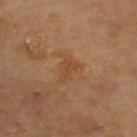Case summary:
– notes: no biopsy performed (imaged during a skin exam)
– image: 15 mm crop, total-body photography
– body site: the upper back
– automated lesion analysis: an area of roughly 3 mm²
– diameter: ≈2.5 mm